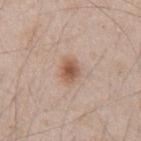Q: Was a biopsy performed?
A: catalogued during a skin exam; not biopsied
Q: What are the patient's age and sex?
A: male, roughly 45 years of age
Q: Lesion location?
A: the abdomen
Q: How was this image acquired?
A: ~15 mm tile from a whole-body skin photo